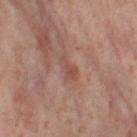Clinical impression: This lesion was catalogued during total-body skin photography and was not selected for biopsy. Background: The recorded lesion diameter is about 3 mm. A female patient, aged 53 to 57. Cropped from a whole-body photographic skin survey; the tile spans about 15 mm. The lesion is on the left thigh. Imaged with cross-polarized lighting.